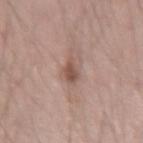Impression:
Imaged during a routine full-body skin examination; the lesion was not biopsied and no histopathology is available.
Background:
A 15 mm crop from a total-body photograph taken for skin-cancer surveillance. A male patient, in their mid- to late 30s. About 3 mm across. The lesion is located on the right upper arm. This is a white-light tile.